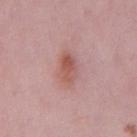<tbp_lesion>
<biopsy_status>not biopsied; imaged during a skin examination</biopsy_status>
<lesion_size>
  <long_diameter_mm_approx>3.5</long_diameter_mm_approx>
</lesion_size>
<lighting>white-light</lighting>
<patient>
  <sex>male</sex>
  <age_approx>50</age_approx>
</patient>
<site>mid back</site>
<image>
  <source>total-body photography crop</source>
  <field_of_view_mm>15</field_of_view_mm>
</image>
<automated_metrics>
  <vs_skin_darker_L>9.0</vs_skin_darker_L>
  <vs_skin_contrast_norm>7.0</vs_skin_contrast_norm>
</automated_metrics>
</tbp_lesion>